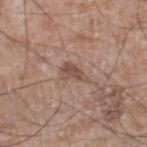Case summary:
• biopsy status: total-body-photography surveillance lesion; no biopsy
• patient: male, in their 60s
• image: total-body-photography crop, ~15 mm field of view
• location: the right lower leg
• image-analysis metrics: a lesion area of about 4.5 mm² and a shape eccentricity near 0.85; an automated nevus-likeness rating near 0 out of 100 and lesion-presence confidence of about 100/100